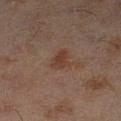Q: Is there a histopathology result?
A: no biopsy performed (imaged during a skin exam)
Q: How was this image acquired?
A: total-body-photography crop, ~15 mm field of view
Q: Where on the body is the lesion?
A: the left lower leg
Q: What are the patient's age and sex?
A: male, aged 43–47